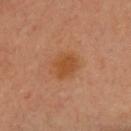Part of a total-body skin-imaging series; this lesion was reviewed on a skin check and was not flagged for biopsy.
A female patient, aged 48 to 52.
Measured at roughly 3.5 mm in maximum diameter.
This is a cross-polarized tile.
A 15 mm close-up tile from a total-body photography series done for melanoma screening.